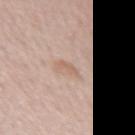biopsy status: imaged on a skin check; not biopsied
tile lighting: white-light illumination
site: the left forearm
automated lesion analysis: a mean CIELAB color near L≈62 a*≈17 b*≈27, a lesion–skin lightness drop of about 7, and a normalized border contrast of about 5
patient: female, about 35 years old
imaging modality: total-body-photography crop, ~15 mm field of view